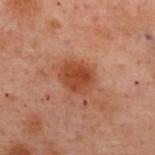• notes · total-body-photography surveillance lesion; no biopsy
• subject · female, aged 53–57
• location · the upper back
• image source · ~15 mm tile from a whole-body skin photo
• automated metrics · a classifier nevus-likeness of about 95/100